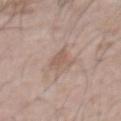The lesion was tiled from a total-body skin photograph and was not biopsied. A male subject aged around 70. A roughly 15 mm field-of-view crop from a total-body skin photograph. The total-body-photography lesion software estimated an area of roughly 4.5 mm². From the chest. Imaged with white-light lighting.